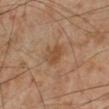A region of skin cropped from a whole-body photographic capture, roughly 15 mm wide. Located on the left lower leg. The lesion's longest dimension is about 3 mm. An algorithmic analysis of the crop reported an area of roughly 6 mm², an eccentricity of roughly 0.7, and a shape-asymmetry score of about 0.2 (0 = symmetric). It also reported a lesion–skin lightness drop of about 8 and a lesion-to-skin contrast of about 6.5 (normalized; higher = more distinct). It also reported a lesion-detection confidence of about 100/100. Imaged with cross-polarized lighting. The subject is a male in their mid- to late 50s.